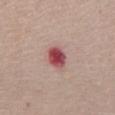Notes:
- workup — total-body-photography surveillance lesion; no biopsy
- patient — female, aged 63–67
- body site — the abdomen
- tile lighting — white-light illumination
- image-analysis metrics — a footprint of about 6 mm² and a symmetry-axis asymmetry near 0.15; an average lesion color of about L≈49 a*≈32 b*≈21 (CIELAB) and a normalized lesion–skin contrast near 11.5; a border-irregularity index near 1.5/10 and a peripheral color-asymmetry measure near 1.5
- lesion size — ~3 mm (longest diameter)
- image — ~15 mm tile from a whole-body skin photo